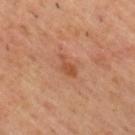Assessment: Captured during whole-body skin photography for melanoma surveillance; the lesion was not biopsied. Acquisition and patient details: A region of skin cropped from a whole-body photographic capture, roughly 15 mm wide. The patient is a male about 55 years old. On the upper back.